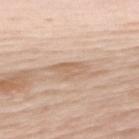biopsy status: imaged on a skin check; not biopsied
TBP lesion metrics: a lesion color around L≈63 a*≈17 b*≈31 in CIELAB, about 8 CIELAB-L* units darker than the surrounding skin, and a normalized border contrast of about 5.5; border irregularity of about 3.5 on a 0–10 scale and peripheral color asymmetry of about 1; a classifier nevus-likeness of about 0/100 and a lesion-detection confidence of about 95/100
patient: female, aged 48–52
lighting: white-light illumination
site: the upper back
image source: 15 mm crop, total-body photography